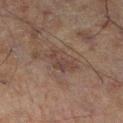notes = total-body-photography surveillance lesion; no biopsy
image = total-body-photography crop, ~15 mm field of view
location = the left leg
patient = male, approximately 60 years of age